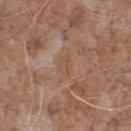The lesion was tiled from a total-body skin photograph and was not biopsied.
The recorded lesion diameter is about 2.5 mm.
This is a white-light tile.
A lesion tile, about 15 mm wide, cut from a 3D total-body photograph.
The lesion is located on the chest.
A male patient, about 70 years old.